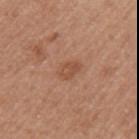Captured during whole-body skin photography for melanoma surveillance; the lesion was not biopsied. Automated image analysis of the tile measured a lesion area of about 4 mm² and an eccentricity of roughly 0.75. The software also gave a mean CIELAB color near L≈51 a*≈23 b*≈32, about 8 CIELAB-L* units darker than the surrounding skin, and a lesion-to-skin contrast of about 5.5 (normalized; higher = more distinct). A 15 mm close-up extracted from a 3D total-body photography capture. On the left upper arm. This is a white-light tile. A female subject, aged 48 to 52. The lesion's longest dimension is about 2.5 mm.